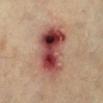The lesion was tiled from a total-body skin photograph and was not biopsied. The patient is approximately 60 years of age. A roughly 15 mm field-of-view crop from a total-body skin photograph. Measured at roughly 8 mm in maximum diameter. The lesion is located on the leg.